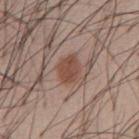This lesion was catalogued during total-body skin photography and was not selected for biopsy. A male subject aged around 55. The lesion is on the abdomen. About 3 mm across. A 15 mm close-up tile from a total-body photography series done for melanoma screening. Automated tile analysis of the lesion measured internal color variation of about 2 on a 0–10 scale. And it measured a nevus-likeness score of about 95/100 and a lesion-detection confidence of about 100/100.